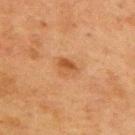Part of a total-body skin-imaging series; this lesion was reviewed on a skin check and was not flagged for biopsy.
Located on the mid back.
Longest diameter approximately 2.5 mm.
Cropped from a whole-body photographic skin survey; the tile spans about 15 mm.
A male patient aged approximately 75.